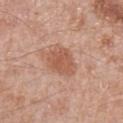{"biopsy_status": "not biopsied; imaged during a skin examination", "lesion_size": {"long_diameter_mm_approx": 4.0}, "site": "left forearm", "automated_metrics": {"area_mm2_approx": 8.5, "eccentricity": 0.65, "shape_asymmetry": 0.25, "border_irregularity_0_10": 3.0, "color_variation_0_10": 2.5, "peripheral_color_asymmetry": 1.0, "nevus_likeness_0_100": 55, "lesion_detection_confidence_0_100": 100}, "patient": {"sex": "female", "age_approx": 50}, "lighting": "white-light", "image": {"source": "total-body photography crop", "field_of_view_mm": 15}}